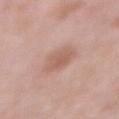Impression: Captured during whole-body skin photography for melanoma surveillance; the lesion was not biopsied. Background: A 15 mm close-up tile from a total-body photography series done for melanoma screening. The lesion is on the front of the torso. A male subject, approximately 55 years of age.